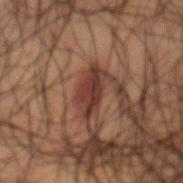Assessment:
Part of a total-body skin-imaging series; this lesion was reviewed on a skin check and was not flagged for biopsy.
Background:
A male patient approximately 50 years of age. Cropped from a total-body skin-imaging series; the visible field is about 15 mm. The lesion is located on the mid back.